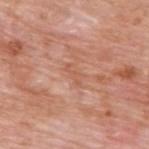workup=no biopsy performed (imaged during a skin exam)
automated metrics=an area of roughly 2.5 mm² and an eccentricity of roughly 0.9; internal color variation of about 0 on a 0–10 scale and peripheral color asymmetry of about 0
illumination=white-light illumination
acquisition=~15 mm tile from a whole-body skin photo
subject=male, aged 58 to 62
anatomic site=the upper back
lesion size=≈2.5 mm